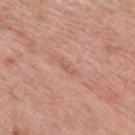No biopsy was performed on this lesion — it was imaged during a full skin examination and was not determined to be concerning.
This is a white-light tile.
Cropped from a total-body skin-imaging series; the visible field is about 15 mm.
A female subject, aged 58–62.
Measured at roughly 2.5 mm in maximum diameter.
Located on the upper back.
Automated tile analysis of the lesion measured an outline eccentricity of about 0.95 (0 = round, 1 = elongated) and two-axis asymmetry of about 0.5. The analysis additionally found a mean CIELAB color near L≈58 a*≈25 b*≈29, a lesion–skin lightness drop of about 7, and a normalized lesion–skin contrast near 4.5. And it measured an automated nevus-likeness rating near 0 out of 100 and a detector confidence of about 100 out of 100 that the crop contains a lesion.